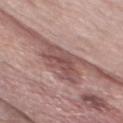{"biopsy_status": "not biopsied; imaged during a skin examination", "patient": {"sex": "male", "age_approx": 55}, "automated_metrics": {"area_mm2_approx": 9.5, "eccentricity": 0.9, "cielab_L": 49, "cielab_a": 21, "cielab_b": 21, "vs_skin_darker_L": 11.0, "vs_skin_contrast_norm": 8.0, "border_irregularity_0_10": 5.0, "color_variation_0_10": 4.0}, "lighting": "white-light", "site": "chest", "image": {"source": "total-body photography crop", "field_of_view_mm": 15}}